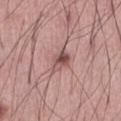* follow-up · imaged on a skin check; not biopsied
* tile lighting · white-light
* automated metrics · a symmetry-axis asymmetry near 0.3; a lesion color around L≈53 a*≈21 b*≈22 in CIELAB, a lesion–skin lightness drop of about 11, and a normalized lesion–skin contrast near 7.5; a color-variation rating of about 8/10 and radial color variation of about 3; a nevus-likeness score of about 75/100 and lesion-presence confidence of about 100/100
* diameter · ~3.5 mm (longest diameter)
* body site · the abdomen
* acquisition · ~15 mm tile from a whole-body skin photo
* patient · male, aged approximately 55